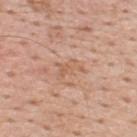Recorded during total-body skin imaging; not selected for excision or biopsy. About 3 mm across. The lesion is on the back. Automated tile analysis of the lesion measured a lesion area of about 4 mm² and a shape eccentricity near 0.8. The analysis additionally found about 7 CIELAB-L* units darker than the surrounding skin. The analysis additionally found border irregularity of about 5.5 on a 0–10 scale, a within-lesion color-variation index near 1.5/10, and radial color variation of about 0.5. The software also gave an automated nevus-likeness rating near 0 out of 100 and lesion-presence confidence of about 95/100. Cropped from a total-body skin-imaging series; the visible field is about 15 mm. Imaged with white-light lighting. The subject is a male about 55 years old.